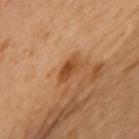Captured under cross-polarized illumination.
From the front of the torso.
A female subject aged around 60.
A region of skin cropped from a whole-body photographic capture, roughly 15 mm wide.